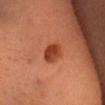Impression: Captured during whole-body skin photography for melanoma surveillance; the lesion was not biopsied. Clinical summary: Cropped from a whole-body photographic skin survey; the tile spans about 15 mm. This is a cross-polarized tile. Automated tile analysis of the lesion measured a border-irregularity rating of about 1.5/10 and a within-lesion color-variation index near 3.5/10. It also reported an automated nevus-likeness rating near 100 out of 100. A female subject roughly 40 years of age. The lesion is located on the head or neck. Longest diameter approximately 3 mm.